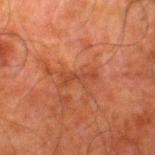Impression:
The lesion was tiled from a total-body skin photograph and was not biopsied.
Clinical summary:
Captured under cross-polarized illumination. The lesion's longest dimension is about 4 mm. The total-body-photography lesion software estimated internal color variation of about 0 on a 0–10 scale. The analysis additionally found a nevus-likeness score of about 0/100 and lesion-presence confidence of about 95/100. A 15 mm close-up extracted from a 3D total-body photography capture. A male subject, about 80 years old. On the leg.